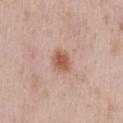{
  "biopsy_status": "not biopsied; imaged during a skin examination",
  "patient": {
    "sex": "male",
    "age_approx": 40
  },
  "site": "chest",
  "lesion_size": {
    "long_diameter_mm_approx": 3.0
  },
  "image": {
    "source": "total-body photography crop",
    "field_of_view_mm": 15
  }
}